A roughly 15 mm field-of-view crop from a total-body skin photograph; from the left upper arm; a female patient, aged around 40:
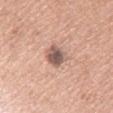The lesion's longest dimension is about 3 mm.
The tile uses white-light illumination.
The biopsy diagnosis was a dysplastic (Clark) nevus.